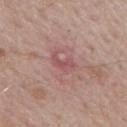Case summary:
– workup — total-body-photography surveillance lesion; no biopsy
– subject — male, aged around 65
– size — about 2.5 mm
– image — ~15 mm tile from a whole-body skin photo
– body site — the mid back